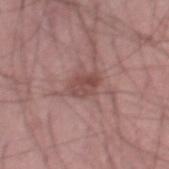biopsy_status: not biopsied; imaged during a skin examination
automated_metrics:
  cielab_L: 48
  cielab_a: 22
  cielab_b: 21
  vs_skin_darker_L: 9.0
  vs_skin_contrast_norm: 7.0
lesion_size:
  long_diameter_mm_approx: 3.0
image:
  source: total-body photography crop
  field_of_view_mm: 15
patient:
  sex: male
  age_approx: 45
site: left thigh
lighting: white-light